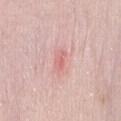Clinical summary: A female patient in their 30s. About 3 mm across. Captured under white-light illumination. The lesion is located on the abdomen. This image is a 15 mm lesion crop taken from a total-body photograph.